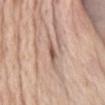Recorded during total-body skin imaging; not selected for excision or biopsy.